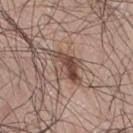Q: Where on the body is the lesion?
A: the lower back
Q: How large is the lesion?
A: ~5 mm (longest diameter)
Q: Illumination type?
A: white-light
Q: Patient demographics?
A: male, aged 68–72
Q: What kind of image is this?
A: ~15 mm tile from a whole-body skin photo
Q: What did automated image analysis measure?
A: a lesion area of about 9.5 mm² and two-axis asymmetry of about 0.3; a border-irregularity rating of about 5/10, internal color variation of about 8.5 on a 0–10 scale, and a peripheral color-asymmetry measure near 3.5; a nevus-likeness score of about 85/100